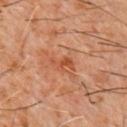The lesion was tiled from a total-body skin photograph and was not biopsied. The lesion is on the chest. A 15 mm crop from a total-body photograph taken for skin-cancer surveillance. The subject is a male aged around 60. Measured at roughly 3 mm in maximum diameter. This is a cross-polarized tile. Automated tile analysis of the lesion measured a lesion–skin lightness drop of about 8 and a normalized border contrast of about 6.5. It also reported a nevus-likeness score of about 0/100 and lesion-presence confidence of about 100/100.